A male subject approximately 30 years of age. On the upper back. Cropped from a whole-body photographic skin survey; the tile spans about 15 mm.
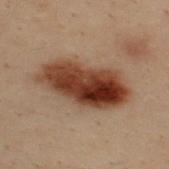Captured under cross-polarized illumination. The biopsy diagnosis was a dysplastic (Clark) nevus — a benign lesion.